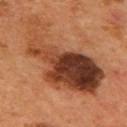Case summary:
– follow-up: imaged on a skin check; not biopsied
– subject: male, about 55 years old
– image-analysis metrics: a lesion color around L≈38 a*≈23 b*≈31 in CIELAB, roughly 15 lightness units darker than nearby skin, and a normalized lesion–skin contrast near 11.5; a nevus-likeness score of about 0/100 and a lesion-detection confidence of about 100/100
– imaging modality: total-body-photography crop, ~15 mm field of view
– body site: the back
– diameter: ~12 mm (longest diameter)
– lighting: cross-polarized